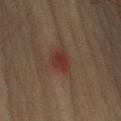<tbp_lesion>
<biopsy_status>not biopsied; imaged during a skin examination</biopsy_status>
<image>
  <source>total-body photography crop</source>
  <field_of_view_mm>15</field_of_view_mm>
</image>
<site>left upper arm</site>
<automated_metrics>
  <area_mm2_approx>5.0</area_mm2_approx>
  <eccentricity>0.5</eccentricity>
  <shape_asymmetry>0.25</shape_asymmetry>
  <vs_skin_contrast_norm>7.0</vs_skin_contrast_norm>
  <lesion_detection_confidence_0_100>100</lesion_detection_confidence_0_100>
</automated_metrics>
<lighting>cross-polarized</lighting>
<patient>
  <sex>male</sex>
  <age_approx>75</age_approx>
</patient>
</tbp_lesion>